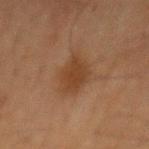<case>
  <biopsy_status>not biopsied; imaged during a skin examination</biopsy_status>
  <patient>
    <sex>male</sex>
    <age_approx>65</age_approx>
  </patient>
  <image>
    <source>total-body photography crop</source>
    <field_of_view_mm>15</field_of_view_mm>
  </image>
  <site>back</site>
  <lesion_size>
    <long_diameter_mm_approx>4.0</long_diameter_mm_approx>
  </lesion_size>
</case>